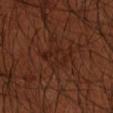This lesion was catalogued during total-body skin photography and was not selected for biopsy. On the left arm. A 15 mm crop from a total-body photograph taken for skin-cancer surveillance. A male subject, in their 50s. The recorded lesion diameter is about 4 mm.